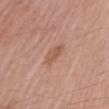Background: The lesion is on the left upper arm. A 15 mm close-up tile from a total-body photography series done for melanoma screening. Approximately 2.5 mm at its widest. A male patient, aged 58 to 62. Captured under white-light illumination.